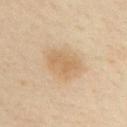{"biopsy_status": "not biopsied; imaged during a skin examination", "image": {"source": "total-body photography crop", "field_of_view_mm": 15}, "patient": {"sex": "male", "age_approx": 50}, "site": "left upper arm"}